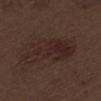Assessment: Recorded during total-body skin imaging; not selected for excision or biopsy. Context: A 15 mm close-up extracted from a 3D total-body photography capture. Imaged with white-light lighting. A male patient, aged approximately 70. Automated image analysis of the tile measured a footprint of about 22 mm², an outline eccentricity of about 0.85 (0 = round, 1 = elongated), and a shape-asymmetry score of about 0.35 (0 = symmetric). The software also gave an average lesion color of about L≈24 a*≈15 b*≈18 (CIELAB), a lesion–skin lightness drop of about 5, and a normalized border contrast of about 6.5. And it measured a lesion-detection confidence of about 100/100. The lesion is on the abdomen.